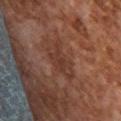The lesion was tiled from a total-body skin photograph and was not biopsied. Captured under cross-polarized illumination. From the upper back. A lesion tile, about 15 mm wide, cut from a 3D total-body photograph. About 5 mm across. A female subject, aged 58–62.